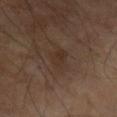No biopsy was performed on this lesion — it was imaged during a full skin examination and was not determined to be concerning. A male patient in their mid-60s. The lesion is on the left arm. Cropped from a whole-body photographic skin survey; the tile spans about 15 mm.